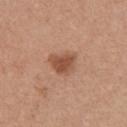size=~3.5 mm (longest diameter)
patient=female, aged 38 to 42
image-analysis metrics=an eccentricity of roughly 0.55 and a symmetry-axis asymmetry near 0.35; a lesion color around L≈51 a*≈23 b*≈31 in CIELAB and a lesion–skin lightness drop of about 11; a nevus-likeness score of about 90/100 and lesion-presence confidence of about 100/100
location=the chest
tile lighting=white-light
imaging modality=15 mm crop, total-body photography